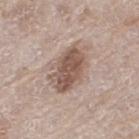{"biopsy_status": "not biopsied; imaged during a skin examination", "automated_metrics": {"area_mm2_approx": 15.0, "eccentricity": 0.8, "shape_asymmetry": 0.2, "vs_skin_darker_L": 13.0, "vs_skin_contrast_norm": 8.5, "border_irregularity_0_10": 2.5, "color_variation_0_10": 4.5}, "image": {"source": "total-body photography crop", "field_of_view_mm": 15}, "lesion_size": {"long_diameter_mm_approx": 5.5}, "site": "left thigh", "patient": {"sex": "female", "age_approx": 75}}